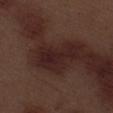Impression: The lesion was tiled from a total-body skin photograph and was not biopsied. Clinical summary: A male subject, in their 70s. The lesion is located on the leg. A close-up tile cropped from a whole-body skin photograph, about 15 mm across.